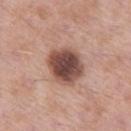The lesion was tiled from a total-body skin photograph and was not biopsied.
A region of skin cropped from a whole-body photographic capture, roughly 15 mm wide.
From the left thigh.
A male patient, aged 53 to 57.
The tile uses white-light illumination.
The lesion's longest dimension is about 5 mm.